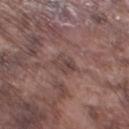biopsy status — catalogued during a skin exam; not biopsied
lighting — white-light illumination
subject — male, approximately 75 years of age
acquisition — ~15 mm crop, total-body skin-cancer survey
size — ~2.5 mm (longest diameter)
body site — the left thigh